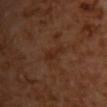<lesion>
  <biopsy_status>not biopsied; imaged during a skin examination</biopsy_status>
  <patient>
    <sex>female</sex>
  </patient>
  <lighting>cross-polarized</lighting>
  <site>upper back</site>
  <automated_metrics>
    <nevus_likeness_0_100>0</nevus_likeness_0_100>
    <lesion_detection_confidence_0_100>100</lesion_detection_confidence_0_100>
  </automated_metrics>
  <image>
    <source>total-body photography crop</source>
    <field_of_view_mm>15</field_of_view_mm>
  </image>
</lesion>